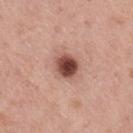| feature | finding |
|---|---|
| workup | catalogued during a skin exam; not biopsied |
| diameter | ~3 mm (longest diameter) |
| patient | male, in their 40s |
| acquisition | 15 mm crop, total-body photography |
| location | the back |